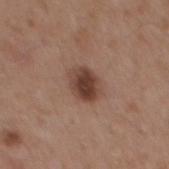{
  "biopsy_status": "not biopsied; imaged during a skin examination",
  "lesion_size": {
    "long_diameter_mm_approx": 3.5
  },
  "site": "mid back",
  "image": {
    "source": "total-body photography crop",
    "field_of_view_mm": 15
  },
  "patient": {
    "sex": "male",
    "age_approx": 55
  }
}